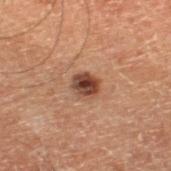{
  "biopsy_status": "not biopsied; imaged during a skin examination",
  "image": {
    "source": "total-body photography crop",
    "field_of_view_mm": 15
  },
  "lesion_size": {
    "long_diameter_mm_approx": 3.0
  },
  "site": "right lower leg",
  "patient": {
    "sex": "male",
    "age_approx": 60
  },
  "lighting": "cross-polarized"
}